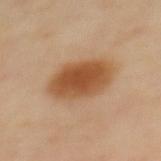Clinical impression:
This lesion was catalogued during total-body skin photography and was not selected for biopsy.
Context:
On the upper back. The patient is a female roughly 45 years of age. The total-body-photography lesion software estimated a footprint of about 19 mm², a shape eccentricity near 0.8, and two-axis asymmetry of about 0.1. It also reported a border-irregularity index near 1.5/10, a within-lesion color-variation index near 4.5/10, and peripheral color asymmetry of about 1. And it measured an automated nevus-likeness rating near 100 out of 100 and a detector confidence of about 100 out of 100 that the crop contains a lesion. The tile uses cross-polarized illumination. Cropped from a total-body skin-imaging series; the visible field is about 15 mm. Measured at roughly 6 mm in maximum diameter.